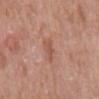Captured during whole-body skin photography for melanoma surveillance; the lesion was not biopsied.
A roughly 15 mm field-of-view crop from a total-body skin photograph.
A male patient, about 65 years old.
Located on the mid back.